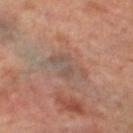Case summary:
* notes: catalogued during a skin exam; not biopsied
* lesion diameter: ~4.5 mm (longest diameter)
* image-analysis metrics: an outline eccentricity of about 0.85 (0 = round, 1 = elongated) and a symmetry-axis asymmetry near 0.4; an average lesion color of about L≈49 a*≈15 b*≈24 (CIELAB), a lesion–skin lightness drop of about 6, and a normalized border contrast of about 5
* acquisition: ~15 mm crop, total-body skin-cancer survey
* location: the left leg
* illumination: cross-polarized
* subject: female, aged 63 to 67